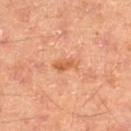follow-up: total-body-photography surveillance lesion; no biopsy | diameter: about 3 mm | image source: total-body-photography crop, ~15 mm field of view | body site: the right thigh | subject: male, aged 63 to 67.